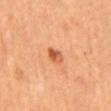Findings:
- follow-up — no biopsy performed (imaged during a skin exam)